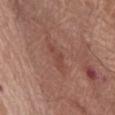No biopsy was performed on this lesion — it was imaged during a full skin examination and was not determined to be concerning.
The lesion's longest dimension is about 3.5 mm.
The patient is a female in their mid-70s.
The lesion is on the left thigh.
The total-body-photography lesion software estimated an outline eccentricity of about 0.95 (0 = round, 1 = elongated) and a symmetry-axis asymmetry near 0.35. And it measured an average lesion color of about L≈45 a*≈24 b*≈26 (CIELAB), roughly 6 lightness units darker than nearby skin, and a normalized border contrast of about 5.5. The analysis additionally found a within-lesion color-variation index near 0/10 and a peripheral color-asymmetry measure near 0. The analysis additionally found an automated nevus-likeness rating near 0 out of 100 and a lesion-detection confidence of about 100/100.
The tile uses white-light illumination.
A close-up tile cropped from a whole-body skin photograph, about 15 mm across.